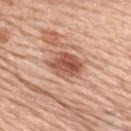| feature | finding |
|---|---|
| workup | no biopsy performed (imaged during a skin exam) |
| diameter | about 4.5 mm |
| image | ~15 mm crop, total-body skin-cancer survey |
| site | the upper back |
| patient | female, aged approximately 65 |